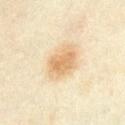Part of a total-body skin-imaging series; this lesion was reviewed on a skin check and was not flagged for biopsy. On the abdomen. This is a cross-polarized tile. About 4.5 mm across. A female subject aged approximately 35. Cropped from a whole-body photographic skin survey; the tile spans about 15 mm.